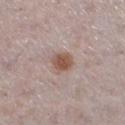follow-up: catalogued during a skin exam; not biopsied
diameter: about 2.5 mm
acquisition: ~15 mm crop, total-body skin-cancer survey
lighting: white-light
anatomic site: the left lower leg
TBP lesion metrics: an eccentricity of roughly 0.4 and a shape-asymmetry score of about 0.25 (0 = symmetric); an automated nevus-likeness rating near 95 out of 100 and a detector confidence of about 100 out of 100 that the crop contains a lesion
subject: female, aged 28–32